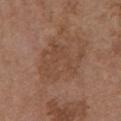patient = female, aged approximately 65
body site = the chest
automated lesion analysis = internal color variation of about 2.5 on a 0–10 scale
tile lighting = white-light
imaging modality = ~15 mm crop, total-body skin-cancer survey
lesion size = about 6 mm